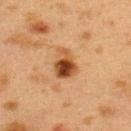Part of a total-body skin-imaging series; this lesion was reviewed on a skin check and was not flagged for biopsy. Cropped from a total-body skin-imaging series; the visible field is about 15 mm. The tile uses cross-polarized illumination. The lesion is located on the upper back. Measured at roughly 3.5 mm in maximum diameter. The total-body-photography lesion software estimated a lesion area of about 8 mm², an eccentricity of roughly 0.7, and a shape-asymmetry score of about 0.35 (0 = symmetric). The software also gave a mean CIELAB color near L≈40 a*≈21 b*≈34 and about 13 CIELAB-L* units darker than the surrounding skin. A female subject, in their 40s.